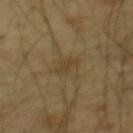Impression: No biopsy was performed on this lesion — it was imaged during a full skin examination and was not determined to be concerning. Image and clinical context: This is a cross-polarized tile. The lesion's longest dimension is about 2.5 mm. A male subject, in their mid- to late 60s. The lesion is located on the mid back. A region of skin cropped from a whole-body photographic capture, roughly 15 mm wide.